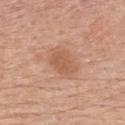Clinical impression:
Recorded during total-body skin imaging; not selected for excision or biopsy.
Context:
The tile uses white-light illumination. A female patient approximately 65 years of age. Measured at roughly 3.5 mm in maximum diameter. A lesion tile, about 15 mm wide, cut from a 3D total-body photograph. The lesion is on the upper back.